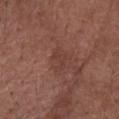| key | value |
|---|---|
| workup | total-body-photography surveillance lesion; no biopsy |
| subject | female, approximately 75 years of age |
| site | the head or neck |
| automated metrics | an area of roughly 3 mm², an outline eccentricity of about 0.8 (0 = round, 1 = elongated), and a shape-asymmetry score of about 0.5 (0 = symmetric); a lesion color around L≈39 a*≈22 b*≈24 in CIELAB and a lesion–skin lightness drop of about 5; a border-irregularity index near 5.5/10, a within-lesion color-variation index near 0/10, and a peripheral color-asymmetry measure near 0; a classifier nevus-likeness of about 0/100 |
| imaging modality | ~15 mm crop, total-body skin-cancer survey |